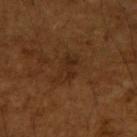Impression:
Imaged during a routine full-body skin examination; the lesion was not biopsied and no histopathology is available.
Acquisition and patient details:
A close-up tile cropped from a whole-body skin photograph, about 15 mm across. A male subject aged 63 to 67. Longest diameter approximately 3.5 mm. Located on the right upper arm.